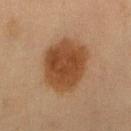Clinical impression:
This lesion was catalogued during total-body skin photography and was not selected for biopsy.
Clinical summary:
The patient is a female roughly 50 years of age. The tile uses cross-polarized illumination. Longest diameter approximately 6.5 mm. A roughly 15 mm field-of-view crop from a total-body skin photograph. Automated image analysis of the tile measured a border-irregularity rating of about 1.5/10, internal color variation of about 3 on a 0–10 scale, and radial color variation of about 1. And it measured an automated nevus-likeness rating near 100 out of 100. From the right thigh.